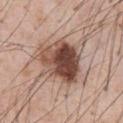Case summary:
- workup: total-body-photography surveillance lesion; no biopsy
- imaging modality: ~15 mm tile from a whole-body skin photo
- automated metrics: an automated nevus-likeness rating near 70 out of 100 and a lesion-detection confidence of about 100/100
- patient: male, aged 53–57
- illumination: white-light
- location: the abdomen
- lesion diameter: about 5.5 mm